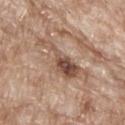<lesion>
<biopsy_status>not biopsied; imaged during a skin examination</biopsy_status>
</lesion>